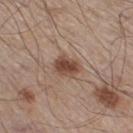The lesion was photographed on a routine skin check and not biopsied; there is no pathology result.
A male patient, aged 58 to 62.
Measured at roughly 3 mm in maximum diameter.
The tile uses white-light illumination.
Located on the right thigh.
Automated image analysis of the tile measured an average lesion color of about L≈46 a*≈19 b*≈27 (CIELAB) and about 13 CIELAB-L* units darker than the surrounding skin. The analysis additionally found an automated nevus-likeness rating near 95 out of 100 and lesion-presence confidence of about 100/100.
This image is a 15 mm lesion crop taken from a total-body photograph.